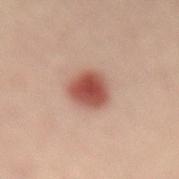Assessment: Part of a total-body skin-imaging series; this lesion was reviewed on a skin check and was not flagged for biopsy. Background: About 4 mm across. Located on the lower back. Cropped from a whole-body photographic skin survey; the tile spans about 15 mm. An algorithmic analysis of the crop reported a footprint of about 11 mm², an eccentricity of roughly 0.45, and a symmetry-axis asymmetry near 0.2. The software also gave an average lesion color of about L≈50 a*≈25 b*≈27 (CIELAB), a lesion–skin lightness drop of about 14, and a normalized border contrast of about 10. The analysis additionally found a border-irregularity rating of about 2/10, a color-variation rating of about 4.5/10, and a peripheral color-asymmetry measure near 1. Captured under cross-polarized illumination. A female patient, aged around 45.